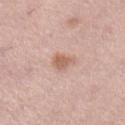  biopsy_status: not biopsied; imaged during a skin examination
  image:
    source: total-body photography crop
    field_of_view_mm: 15
  automated_metrics:
    vs_skin_darker_L: 10.0
    vs_skin_contrast_norm: 7.0
    border_irregularity_0_10: 3.5
    color_variation_0_10: 2.0
    peripheral_color_asymmetry: 1.0
    lesion_detection_confidence_0_100: 100
  site: leg
  lesion_size:
    long_diameter_mm_approx: 3.0
  patient:
    sex: female
    age_approx: 35